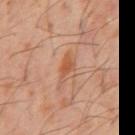The lesion was photographed on a routine skin check and not biopsied; there is no pathology result.
A male subject, aged 58–62.
The lesion-visualizer software estimated a footprint of about 4.5 mm². The software also gave a mean CIELAB color near L≈51 a*≈23 b*≈33, a lesion–skin lightness drop of about 8, and a lesion-to-skin contrast of about 7 (normalized; higher = more distinct). And it measured a border-irregularity rating of about 4.5/10 and peripheral color asymmetry of about 0.5. And it measured a detector confidence of about 100 out of 100 that the crop contains a lesion.
Captured under cross-polarized illumination.
A 15 mm close-up extracted from a 3D total-body photography capture.
On the abdomen.
The recorded lesion diameter is about 3.5 mm.